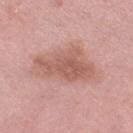Q: Was a biopsy performed?
A: no biopsy performed (imaged during a skin exam)
Q: How was this image acquired?
A: total-body-photography crop, ~15 mm field of view
Q: Where on the body is the lesion?
A: the leg
Q: Patient demographics?
A: female, approximately 70 years of age